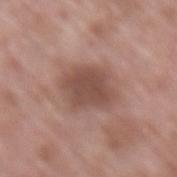| field | value |
|---|---|
| notes | no biopsy performed (imaged during a skin exam) |
| location | the lower back |
| diameter | ≈5 mm |
| subject | male, aged 53–57 |
| lighting | white-light illumination |
| automated lesion analysis | a lesion color around L≈49 a*≈19 b*≈24 in CIELAB and a normalized border contrast of about 7.5; a border-irregularity index near 1.5/10 and radial color variation of about 0.5 |
| imaging modality | ~15 mm tile from a whole-body skin photo |